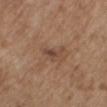<lesion>
<biopsy_status>not biopsied; imaged during a skin examination</biopsy_status>
<image>
  <source>total-body photography crop</source>
  <field_of_view_mm>15</field_of_view_mm>
</image>
<lesion_size>
  <long_diameter_mm_approx>2.5</long_diameter_mm_approx>
</lesion_size>
<site>mid back</site>
<lighting>white-light</lighting>
<patient>
  <sex>female</sex>
  <age_approx>65</age_approx>
</patient>
</lesion>